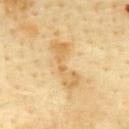{"biopsy_status": "not biopsied; imaged during a skin examination", "automated_metrics": {"area_mm2_approx": 9.5, "eccentricity": 0.95, "shape_asymmetry": 0.45, "cielab_L": 69, "cielab_a": 16, "cielab_b": 45, "vs_skin_darker_L": 9.0, "vs_skin_contrast_norm": 6.0}, "lesion_size": {"long_diameter_mm_approx": 6.0}, "image": {"source": "total-body photography crop", "field_of_view_mm": 15}, "lighting": "cross-polarized", "patient": {"sex": "male", "age_approx": 65}, "site": "chest"}